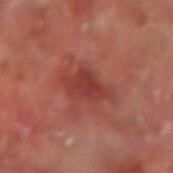Part of a total-body skin-imaging series; this lesion was reviewed on a skin check and was not flagged for biopsy.
A lesion tile, about 15 mm wide, cut from a 3D total-body photograph.
A male subject, in their mid-60s.
The lesion's longest dimension is about 5 mm.
The tile uses cross-polarized illumination.
The lesion is on the left forearm.